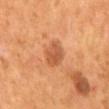  biopsy_status: not biopsied; imaged during a skin examination
  image:
    source: total-body photography crop
    field_of_view_mm: 15
  patient:
    sex: male
    age_approx: 55
  site: mid back